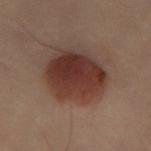<record>
  <biopsy_status>not biopsied; imaged during a skin examination</biopsy_status>
  <automated_metrics>
    <border_irregularity_0_10>1.5</border_irregularity_0_10>
    <color_variation_0_10>5.5</color_variation_0_10>
    <nevus_likeness_0_100>100</nevus_likeness_0_100>
    <lesion_detection_confidence_0_100>100</lesion_detection_confidence_0_100>
  </automated_metrics>
  <image>
    <source>total-body photography crop</source>
    <field_of_view_mm>15</field_of_view_mm>
  </image>
  <site>right leg</site>
  <patient>
    <sex>female</sex>
    <age_approx>60</age_approx>
  </patient>
  <lesion_size>
    <long_diameter_mm_approx>7.0</long_diameter_mm_approx>
  </lesion_size>
</record>